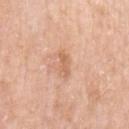Assessment: The lesion was tiled from a total-body skin photograph and was not biopsied. Image and clinical context: A female patient in their mid-60s. Longest diameter approximately 3 mm. Located on the right upper arm. A region of skin cropped from a whole-body photographic capture, roughly 15 mm wide. Captured under white-light illumination.